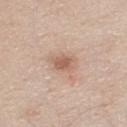Captured during whole-body skin photography for melanoma surveillance; the lesion was not biopsied. On the chest. A close-up tile cropped from a whole-body skin photograph, about 15 mm across. Automated image analysis of the tile measured a border-irregularity rating of about 2.5/10 and a color-variation rating of about 2/10. And it measured a nevus-likeness score of about 60/100 and a detector confidence of about 100 out of 100 that the crop contains a lesion. Measured at roughly 2.5 mm in maximum diameter. A male patient, approximately 45 years of age.